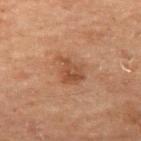Captured during whole-body skin photography for melanoma surveillance; the lesion was not biopsied.
The tile uses cross-polarized illumination.
A male subject aged 68–72.
The lesion is on the left thigh.
A lesion tile, about 15 mm wide, cut from a 3D total-body photograph.
Approximately 4 mm at its widest.